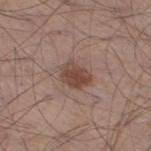{
  "biopsy_status": "not biopsied; imaged during a skin examination",
  "lesion_size": {
    "long_diameter_mm_approx": 3.5
  },
  "patient": {
    "sex": "male",
    "age_approx": 55
  },
  "site": "right thigh",
  "lighting": "white-light",
  "image": {
    "source": "total-body photography crop",
    "field_of_view_mm": 15
  }
}